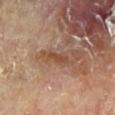This image is a 15 mm lesion crop taken from a total-body photograph.
Located on the left lower leg.
The patient is a male in their mid- to late 80s or older.